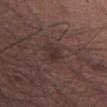Clinical impression: No biopsy was performed on this lesion — it was imaged during a full skin examination and was not determined to be concerning. Context: Captured under white-light illumination. Cropped from a total-body skin-imaging series; the visible field is about 15 mm. The lesion is on the left thigh. The patient is a male aged around 60. Automated image analysis of the tile measured a lesion area of about 4.5 mm², an outline eccentricity of about 0.8 (0 = round, 1 = elongated), and a shape-asymmetry score of about 0.3 (0 = symmetric). The software also gave a border-irregularity rating of about 3/10, a color-variation rating of about 2.5/10, and a peripheral color-asymmetry measure near 1.